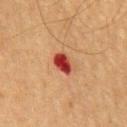<lesion>
  <biopsy_status>not biopsied; imaged during a skin examination</biopsy_status>
  <image>
    <source>total-body photography crop</source>
    <field_of_view_mm>15</field_of_view_mm>
  </image>
  <site>chest</site>
  <patient>
    <sex>male</sex>
    <age_approx>65</age_approx>
  </patient>
  <lesion_size>
    <long_diameter_mm_approx>3.0</long_diameter_mm_approx>
  </lesion_size>
  <lighting>cross-polarized</lighting>
</lesion>